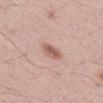Clinical summary:
Measured at roughly 3 mm in maximum diameter. A lesion tile, about 15 mm wide, cut from a 3D total-body photograph. The tile uses white-light illumination. The patient is a male aged 53 to 57. From the front of the torso.